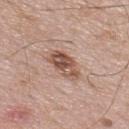This lesion was catalogued during total-body skin photography and was not selected for biopsy. A male subject, aged around 70. A close-up tile cropped from a whole-body skin photograph, about 15 mm across. Measured at roughly 4 mm in maximum diameter. From the upper back. Imaged with white-light lighting. The lesion-visualizer software estimated a footprint of about 8.5 mm² and a shape eccentricity near 0.8.